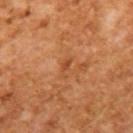{"patient": {"sex": "male", "age_approx": 65}, "lighting": "cross-polarized", "automated_metrics": {"area_mm2_approx": 2.0, "eccentricity": 0.9, "cielab_L": 48, "cielab_a": 27, "cielab_b": 39, "vs_skin_darker_L": 7.0, "vs_skin_contrast_norm": 5.5, "border_irregularity_0_10": 6.0, "color_variation_0_10": 0.0, "nevus_likeness_0_100": 0, "lesion_detection_confidence_0_100": 100}, "image": {"source": "total-body photography crop", "field_of_view_mm": 15}, "lesion_size": {"long_diameter_mm_approx": 2.5}}